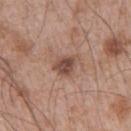Captured during whole-body skin photography for melanoma surveillance; the lesion was not biopsied.
The subject is a male aged approximately 65.
This image is a 15 mm lesion crop taken from a total-body photograph.
About 3 mm across.
From the arm.
This is a white-light tile.
An algorithmic analysis of the crop reported an average lesion color of about L≈48 a*≈19 b*≈26 (CIELAB) and a normalized border contrast of about 8.5. It also reported a color-variation rating of about 3.5/10 and radial color variation of about 1. The analysis additionally found a lesion-detection confidence of about 100/100.